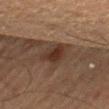diameter: ≈3.5 mm | patient: male, aged 73 to 77 | site: the right thigh | lighting: cross-polarized illumination | image: ~15 mm tile from a whole-body skin photo.